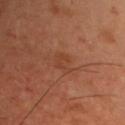<lesion>
<biopsy_status>not biopsied; imaged during a skin examination</biopsy_status>
<site>chest</site>
<lighting>cross-polarized</lighting>
<patient>
  <sex>male</sex>
  <age_approx>40</age_approx>
</patient>
<image>
  <source>total-body photography crop</source>
  <field_of_view_mm>15</field_of_view_mm>
</image>
<automated_metrics>
  <border_irregularity_0_10>6.0</border_irregularity_0_10>
  <color_variation_0_10>0.0</color_variation_0_10>
  <peripheral_color_asymmetry>0.0</peripheral_color_asymmetry>
  <lesion_detection_confidence_0_100>100</lesion_detection_confidence_0_100>
</automated_metrics>
</lesion>